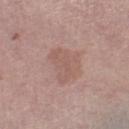notes — total-body-photography surveillance lesion; no biopsy